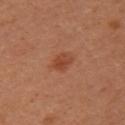| feature | finding |
|---|---|
| subject | female, approximately 30 years of age |
| image source | ~15 mm crop, total-body skin-cancer survey |
| location | the left upper arm |